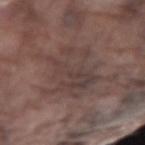{
  "patient": {
    "sex": "male",
    "age_approx": 75
  },
  "automated_metrics": {
    "area_mm2_approx": 21.0,
    "eccentricity": 0.7,
    "shape_asymmetry": 0.35,
    "lesion_detection_confidence_0_100": 55
  },
  "lesion_size": {
    "long_diameter_mm_approx": 7.0
  },
  "image": {
    "source": "total-body photography crop",
    "field_of_view_mm": 15
  },
  "site": "right forearm"
}